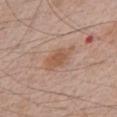- notes: imaged on a skin check; not biopsied